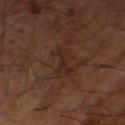Part of a total-body skin-imaging series; this lesion was reviewed on a skin check and was not flagged for biopsy. Automated image analysis of the tile measured a border-irregularity index near 6/10 and a peripheral color-asymmetry measure near 0. The software also gave an automated nevus-likeness rating near 0 out of 100 and a detector confidence of about 80 out of 100 that the crop contains a lesion. The lesion is located on the leg. A close-up tile cropped from a whole-body skin photograph, about 15 mm across. A male subject, in their mid-60s. Measured at roughly 3 mm in maximum diameter.